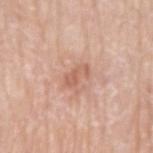Impression: The lesion was photographed on a routine skin check and not biopsied; there is no pathology result. Image and clinical context: About 3.5 mm across. A male patient aged 78–82. A lesion tile, about 15 mm wide, cut from a 3D total-body photograph. The lesion is located on the arm. Imaged with white-light lighting.